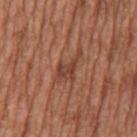| key | value |
|---|---|
| follow-up | imaged on a skin check; not biopsied |
| subject | male, in their mid-60s |
| imaging modality | total-body-photography crop, ~15 mm field of view |
| body site | the mid back |